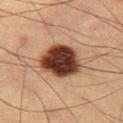biopsy_status: not biopsied; imaged during a skin examination
patient:
  sex: male
  age_approx: 55
site: leg
image:
  source: total-body photography crop
  field_of_view_mm: 15